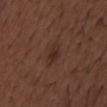No biopsy was performed on this lesion — it was imaged during a full skin examination and was not determined to be concerning. This image is a 15 mm lesion crop taken from a total-body photograph. A male patient, aged 48–52. The tile uses white-light illumination. The lesion's longest dimension is about 4 mm.